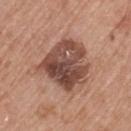– notes: imaged on a skin check; not biopsied
– patient: female, aged 73–77
– image source: 15 mm crop, total-body photography
– body site: the left thigh